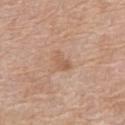{
  "automated_metrics": {
    "eccentricity": 0.75,
    "shape_asymmetry": 0.4,
    "nevus_likeness_0_100": 0,
    "lesion_detection_confidence_0_100": 100
  },
  "site": "front of the torso",
  "image": {
    "source": "total-body photography crop",
    "field_of_view_mm": 15
  },
  "lighting": "white-light",
  "patient": {
    "sex": "female",
    "age_approx": 60
  }
}